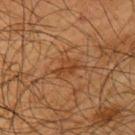Q: Was this lesion biopsied?
A: total-body-photography surveillance lesion; no biopsy
Q: Lesion location?
A: the left upper arm
Q: How was this image acquired?
A: ~15 mm tile from a whole-body skin photo
Q: Who is the patient?
A: male, aged around 65
Q: Illumination type?
A: cross-polarized illumination